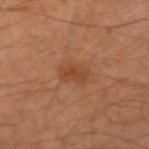follow-up: imaged on a skin check; not biopsied | image: total-body-photography crop, ~15 mm field of view | subject: male, in their mid- to late 40s | location: the left upper arm | automated metrics: a footprint of about 3.5 mm², a shape eccentricity near 0.85, and two-axis asymmetry of about 0.35; an average lesion color of about L≈43 a*≈25 b*≈34 (CIELAB) and about 7 CIELAB-L* units darker than the surrounding skin; border irregularity of about 3.5 on a 0–10 scale, a within-lesion color-variation index near 1.5/10, and peripheral color asymmetry of about 0.5 | tile lighting: cross-polarized | lesion size: ~3 mm (longest diameter).